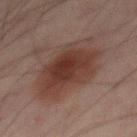– biopsy status: no biopsy performed (imaged during a skin exam)
– tile lighting: cross-polarized illumination
– patient: male, aged 63 to 67
– site: the back
– diameter: ≈8 mm
– imaging modality: ~15 mm crop, total-body skin-cancer survey